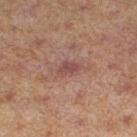A 15 mm close-up extracted from a 3D total-body photography capture.
A male patient in their mid- to late 60s.
Captured under cross-polarized illumination.
The lesion is located on the left lower leg.
An algorithmic analysis of the crop reported a footprint of about 3.5 mm², an eccentricity of roughly 0.8, and two-axis asymmetry of about 0.35. The software also gave border irregularity of about 3.5 on a 0–10 scale, a color-variation rating of about 1.5/10, and a peripheral color-asymmetry measure near 0.5.